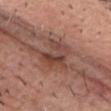A male subject, aged approximately 80.
An algorithmic analysis of the crop reported a footprint of about 12 mm², an eccentricity of roughly 0.55, and a shape-asymmetry score of about 0.4 (0 = symmetric). And it measured border irregularity of about 4.5 on a 0–10 scale and a within-lesion color-variation index near 8/10.
This is a white-light tile.
A region of skin cropped from a whole-body photographic capture, roughly 15 mm wide.
Located on the chest.
About 5 mm across.